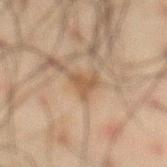{
  "biopsy_status": "not biopsied; imaged during a skin examination",
  "lighting": "cross-polarized",
  "site": "abdomen",
  "lesion_size": {
    "long_diameter_mm_approx": 3.0
  },
  "patient": {
    "sex": "male",
    "age_approx": 60
  },
  "image": {
    "source": "total-body photography crop",
    "field_of_view_mm": 15
  }
}